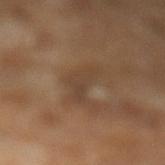Part of a total-body skin-imaging series; this lesion was reviewed on a skin check and was not flagged for biopsy. A lesion tile, about 15 mm wide, cut from a 3D total-body photograph. Captured under cross-polarized illumination. Automated tile analysis of the lesion measured a footprint of about 6.5 mm², a shape eccentricity near 0.9, and two-axis asymmetry of about 0.55. It also reported a mean CIELAB color near L≈40 a*≈15 b*≈27, roughly 6 lightness units darker than nearby skin, and a lesion-to-skin contrast of about 5.5 (normalized; higher = more distinct). It also reported a within-lesion color-variation index near 1.5/10 and peripheral color asymmetry of about 0.5. It also reported a nevus-likeness score of about 0/100. The subject is a male aged around 65.